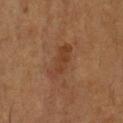notes: catalogued during a skin exam; not biopsied | location: the front of the torso | acquisition: ~15 mm crop, total-body skin-cancer survey | patient: male, about 55 years old.